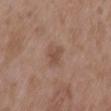Imaged during a routine full-body skin examination; the lesion was not biopsied and no histopathology is available. Captured under white-light illumination. A close-up tile cropped from a whole-body skin photograph, about 15 mm across. Automated image analysis of the tile measured a border-irregularity index near 4/10 and a within-lesion color-variation index near 1.5/10. A female subject aged around 35. Located on the left upper arm.